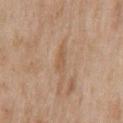Q: Was this lesion biopsied?
A: no biopsy performed (imaged during a skin exam)
Q: Lesion size?
A: ≈2.5 mm
Q: Who is the patient?
A: male, approximately 65 years of age
Q: Where on the body is the lesion?
A: the chest
Q: What kind of image is this?
A: ~15 mm crop, total-body skin-cancer survey
Q: What lighting was used for the tile?
A: white-light illumination